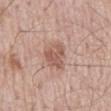{"biopsy_status": "not biopsied; imaged during a skin examination", "lighting": "white-light", "patient": {"sex": "male", "age_approx": 65}, "image": {"source": "total-body photography crop", "field_of_view_mm": 15}, "site": "mid back"}